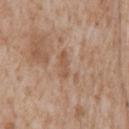No biopsy was performed on this lesion — it was imaged during a full skin examination and was not determined to be concerning. A close-up tile cropped from a whole-body skin photograph, about 15 mm across. A male subject, about 65 years old. On the chest.